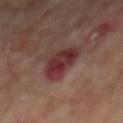Part of a total-body skin-imaging series; this lesion was reviewed on a skin check and was not flagged for biopsy. On the mid back. A 15 mm close-up extracted from a 3D total-body photography capture. A male subject, roughly 65 years of age.